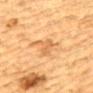Imaged during a routine full-body skin examination; the lesion was not biopsied and no histopathology is available. A 15 mm crop from a total-body photograph taken for skin-cancer surveillance. A male subject, aged 83 or older. Longest diameter approximately 3.5 mm. Imaged with cross-polarized lighting. From the upper back.